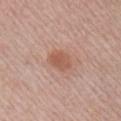| key | value |
|---|---|
| workup | no biopsy performed (imaged during a skin exam) |
| anatomic site | the right upper arm |
| automated lesion analysis | a nevus-likeness score of about 75/100 and lesion-presence confidence of about 100/100 |
| tile lighting | white-light |
| subject | male, approximately 55 years of age |
| imaging modality | total-body-photography crop, ~15 mm field of view |